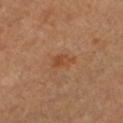| feature | finding |
|---|---|
| site | the left lower leg |
| image source | ~15 mm tile from a whole-body skin photo |
| automated lesion analysis | a lesion area of about 4 mm², an outline eccentricity of about 0.8 (0 = round, 1 = elongated), and two-axis asymmetry of about 0.3; a lesion color around L≈45 a*≈22 b*≈34 in CIELAB, a lesion–skin lightness drop of about 6, and a normalized lesion–skin contrast near 6 |
| lighting | cross-polarized illumination |
| patient | female, aged approximately 50 |
| diameter | ≈2.5 mm |